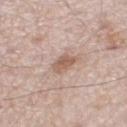Impression:
Imaged during a routine full-body skin examination; the lesion was not biopsied and no histopathology is available.
Image and clinical context:
A close-up tile cropped from a whole-body skin photograph, about 15 mm across. On the left thigh. A male subject, aged around 55.